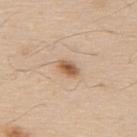The lesion was tiled from a total-body skin photograph and was not biopsied. A male patient, about 60 years old. The lesion is located on the upper back. Measured at roughly 3 mm in maximum diameter. Imaged with white-light lighting. A 15 mm close-up tile from a total-body photography series done for melanoma screening.